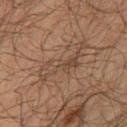This lesion was catalogued during total-body skin photography and was not selected for biopsy.
The lesion is on the right thigh.
A region of skin cropped from a whole-body photographic capture, roughly 15 mm wide.
About 7 mm across.
Automated tile analysis of the lesion measured a lesion area of about 11 mm², an outline eccentricity of about 0.95 (0 = round, 1 = elongated), and two-axis asymmetry of about 0.6.
The subject is a male roughly 65 years of age.